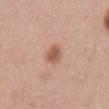The lesion was photographed on a routine skin check and not biopsied; there is no pathology result. The tile uses white-light illumination. A male subject aged 48–52. From the mid back. Cropped from a whole-body photographic skin survey; the tile spans about 15 mm. Longest diameter approximately 3 mm. The total-body-photography lesion software estimated a lesion area of about 5 mm². It also reported border irregularity of about 2 on a 0–10 scale, a within-lesion color-variation index near 3/10, and a peripheral color-asymmetry measure near 1.